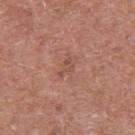Clinical impression: Part of a total-body skin-imaging series; this lesion was reviewed on a skin check and was not flagged for biopsy. Acquisition and patient details: A 15 mm close-up extracted from a 3D total-body photography capture. The patient is a male approximately 75 years of age. Captured under white-light illumination. The lesion is on the arm.